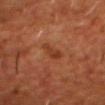<tbp_lesion>
<image>
  <source>total-body photography crop</source>
  <field_of_view_mm>15</field_of_view_mm>
</image>
<site>head or neck</site>
<patient>
  <sex>male</sex>
  <age_approx>55</age_approx>
</patient>
<lighting>cross-polarized</lighting>
</tbp_lesion>